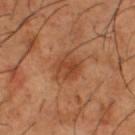Clinical impression: Captured during whole-body skin photography for melanoma surveillance; the lesion was not biopsied. Clinical summary: Measured at roughly 3.5 mm in maximum diameter. The subject is a male aged 63 to 67. Imaged with cross-polarized lighting. A region of skin cropped from a whole-body photographic capture, roughly 15 mm wide. Located on the left thigh.